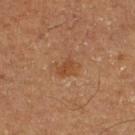Part of a total-body skin-imaging series; this lesion was reviewed on a skin check and was not flagged for biopsy.
A male subject, roughly 65 years of age.
The lesion is located on the leg.
Cropped from a whole-body photographic skin survey; the tile spans about 15 mm.